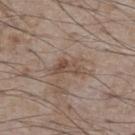{
  "biopsy_status": "not biopsied; imaged during a skin examination",
  "image": {
    "source": "total-body photography crop",
    "field_of_view_mm": 15
  },
  "lesion_size": {
    "long_diameter_mm_approx": 3.5
  },
  "site": "chest",
  "patient": {
    "sex": "male",
    "age_approx": 75
  },
  "lighting": "white-light",
  "automated_metrics": {
    "area_mm2_approx": 5.5,
    "eccentricity": 0.85,
    "shape_asymmetry": 0.35,
    "cielab_L": 48,
    "cielab_a": 15,
    "cielab_b": 25,
    "vs_skin_darker_L": 9.0,
    "nevus_likeness_0_100": 0
  }
}